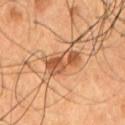biopsy status = imaged on a skin check; not biopsied | location = the chest | image source = 15 mm crop, total-body photography | subject = male, aged 53–57.